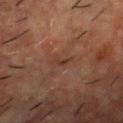Case summary:
• follow-up: no biopsy performed (imaged during a skin exam)
• image: 15 mm crop, total-body photography
• subject: male, approximately 60 years of age
• automated lesion analysis: a lesion color around L≈29 a*≈17 b*≈23 in CIELAB, a lesion–skin lightness drop of about 5, and a normalized lesion–skin contrast near 5.5; border irregularity of about 4.5 on a 0–10 scale and a peripheral color-asymmetry measure near 0; a nevus-likeness score of about 0/100 and a detector confidence of about 90 out of 100 that the crop contains a lesion
• illumination: cross-polarized
• diameter: ≈2.5 mm
• body site: the chest